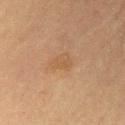The lesion was tiled from a total-body skin photograph and was not biopsied. The subject is a male in their mid- to late 60s. Located on the chest. The lesion-visualizer software estimated a footprint of about 5 mm² and an outline eccentricity of about 0.7 (0 = round, 1 = elongated). It also reported an average lesion color of about L≈44 a*≈15 b*≈30 (CIELAB), about 4 CIELAB-L* units darker than the surrounding skin, and a normalized lesion–skin contrast near 4.5. And it measured border irregularity of about 2.5 on a 0–10 scale and a color-variation rating of about 2/10. A region of skin cropped from a whole-body photographic capture, roughly 15 mm wide.